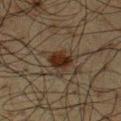Impression: Part of a total-body skin-imaging series; this lesion was reviewed on a skin check and was not flagged for biopsy.